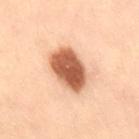– notes — total-body-photography surveillance lesion; no biopsy
– lesion size — ≈5.5 mm
– subject — male, aged approximately 50
– body site — the back
– lighting — cross-polarized illumination
– acquisition — ~15 mm crop, total-body skin-cancer survey